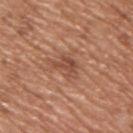Assessment: This lesion was catalogued during total-body skin photography and was not selected for biopsy. Context: A lesion tile, about 15 mm wide, cut from a 3D total-body photograph. A male patient approximately 65 years of age. Located on the upper back.